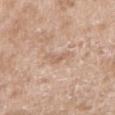Impression:
Imaged during a routine full-body skin examination; the lesion was not biopsied and no histopathology is available.
Background:
The recorded lesion diameter is about 3 mm. The total-body-photography lesion software estimated a lesion–skin lightness drop of about 7 and a normalized border contrast of about 4.5. This is a white-light tile. Located on the right upper arm. A 15 mm crop from a total-body photograph taken for skin-cancer surveillance. The subject is a male roughly 50 years of age.